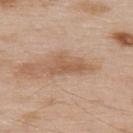{"biopsy_status": "not biopsied; imaged during a skin examination", "site": "upper back", "lighting": "white-light", "image": {"source": "total-body photography crop", "field_of_view_mm": 15}, "automated_metrics": {"area_mm2_approx": 7.0, "eccentricity": 0.9, "shape_asymmetry": 0.35, "border_irregularity_0_10": 5.0, "color_variation_0_10": 2.0, "peripheral_color_asymmetry": 1.0, "lesion_detection_confidence_0_100": 100}, "patient": {"sex": "male", "age_approx": 55}, "lesion_size": {"long_diameter_mm_approx": 5.0}}